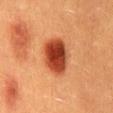workup = total-body-photography surveillance lesion; no biopsy
acquisition = 15 mm crop, total-body photography
anatomic site = the mid back
subject = male, roughly 40 years of age